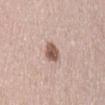* workup: catalogued during a skin exam; not biopsied
* anatomic site: the abdomen
* imaging modality: total-body-photography crop, ~15 mm field of view
* lesion diameter: about 3 mm
* subject: female, about 50 years old
* illumination: white-light illumination